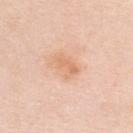Findings:
– biopsy status · total-body-photography surveillance lesion; no biopsy
– size · ~3 mm (longest diameter)
– imaging modality · ~15 mm tile from a whole-body skin photo
– patient · female, aged approximately 45
– illumination · white-light
– anatomic site · the upper back